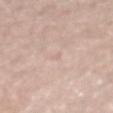Imaged during a routine full-body skin examination; the lesion was not biopsied and no histopathology is available. The patient is a male aged 53–57. The lesion is located on the back. This image is a 15 mm lesion crop taken from a total-body photograph. The total-body-photography lesion software estimated a lesion-to-skin contrast of about 3 (normalized; higher = more distinct). The analysis additionally found a border-irregularity rating of about 2.5/10, internal color variation of about 0 on a 0–10 scale, and radial color variation of about 0. About 1.5 mm across. This is a white-light tile.